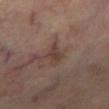biopsy status: total-body-photography surveillance lesion; no biopsy
anatomic site: the left lower leg
automated metrics: an area of roughly 3.5 mm², an outline eccentricity of about 0.8 (0 = round, 1 = elongated), and a shape-asymmetry score of about 0.4 (0 = symmetric); an average lesion color of about L≈37 a*≈16 b*≈21 (CIELAB), a lesion–skin lightness drop of about 7, and a normalized lesion–skin contrast near 6.5; a classifier nevus-likeness of about 0/100 and lesion-presence confidence of about 80/100
lesion diameter: ~3 mm (longest diameter)
acquisition: total-body-photography crop, ~15 mm field of view
lighting: cross-polarized
subject: male, about 70 years old